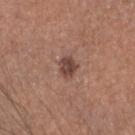No biopsy was performed on this lesion — it was imaged during a full skin examination and was not determined to be concerning. The total-body-photography lesion software estimated an outline eccentricity of about 0.65 (0 = round, 1 = elongated) and a shape-asymmetry score of about 0.25 (0 = symmetric). A 15 mm crop from a total-body photograph taken for skin-cancer surveillance. On the head or neck. The tile uses white-light illumination. About 2.5 mm across. A female patient approximately 30 years of age.